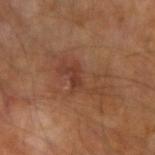Captured during whole-body skin photography for melanoma surveillance; the lesion was not biopsied. An algorithmic analysis of the crop reported an area of roughly 8 mm². The analysis additionally found a mean CIELAB color near L≈38 a*≈22 b*≈28, roughly 7 lightness units darker than nearby skin, and a lesion-to-skin contrast of about 6 (normalized; higher = more distinct). The analysis additionally found a border-irregularity rating of about 9/10 and a peripheral color-asymmetry measure near 0.5. The analysis additionally found a nevus-likeness score of about 0/100. A male patient roughly 65 years of age. Longest diameter approximately 5.5 mm. The lesion is on the left arm. This is a cross-polarized tile. Cropped from a whole-body photographic skin survey; the tile spans about 15 mm.